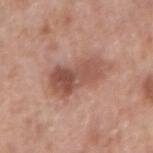| field | value |
|---|---|
| follow-up | no biopsy performed (imaged during a skin exam) |
| patient | male, about 70 years old |
| lesion size | about 7 mm |
| TBP lesion metrics | a lesion area of about 17 mm², a shape eccentricity near 0.9, and a symmetry-axis asymmetry near 0.25; a border-irregularity index near 3/10, internal color variation of about 6.5 on a 0–10 scale, and radial color variation of about 2.5; a classifier nevus-likeness of about 45/100 and lesion-presence confidence of about 100/100 |
| illumination | white-light illumination |
| site | the right upper arm |
| imaging modality | total-body-photography crop, ~15 mm field of view |